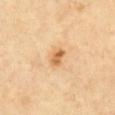| key | value |
|---|---|
| workup | catalogued during a skin exam; not biopsied |
| tile lighting | cross-polarized illumination |
| patient | aged 58 to 62 |
| lesion size | ≈2.5 mm |
| site | the chest |
| image source | total-body-photography crop, ~15 mm field of view |